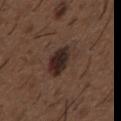This lesion was catalogued during total-body skin photography and was not selected for biopsy.
An algorithmic analysis of the crop reported a mean CIELAB color near L≈26 a*≈15 b*≈19, roughly 11 lightness units darker than nearby skin, and a normalized border contrast of about 12. The software also gave a detector confidence of about 100 out of 100 that the crop contains a lesion.
The lesion's longest dimension is about 4 mm.
Imaged with white-light lighting.
A male subject in their 50s.
A region of skin cropped from a whole-body photographic capture, roughly 15 mm wide.
On the chest.